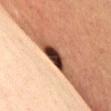This lesion was catalogued during total-body skin photography and was not selected for biopsy.